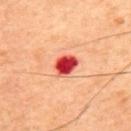Impression:
Recorded during total-body skin imaging; not selected for excision or biopsy.
Image and clinical context:
Longest diameter approximately 3 mm. A roughly 15 mm field-of-view crop from a total-body skin photograph. The lesion-visualizer software estimated a border-irregularity index near 2/10, a within-lesion color-variation index near 5.5/10, and a peripheral color-asymmetry measure near 1.5. Located on the back. The subject is a male about 45 years old. The tile uses cross-polarized illumination.